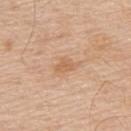Findings:
– notes: catalogued during a skin exam; not biopsied
– patient: male, approximately 80 years of age
– image: ~15 mm tile from a whole-body skin photo
– lesion size: ≈2.5 mm
– image-analysis metrics: a classifier nevus-likeness of about 0/100 and a detector confidence of about 100 out of 100 that the crop contains a lesion
– site: the upper back